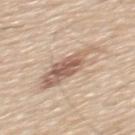Assessment: Captured during whole-body skin photography for melanoma surveillance; the lesion was not biopsied. Image and clinical context: Measured at roughly 7 mm in maximum diameter. Cropped from a total-body skin-imaging series; the visible field is about 15 mm. This is a white-light tile. From the mid back. A male subject, aged around 60. An algorithmic analysis of the crop reported an area of roughly 16 mm², an outline eccentricity of about 0.9 (0 = round, 1 = elongated), and a shape-asymmetry score of about 0.25 (0 = symmetric). And it measured a lesion–skin lightness drop of about 12. And it measured an automated nevus-likeness rating near 95 out of 100.